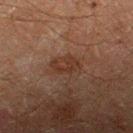Notes:
– workup · imaged on a skin check; not biopsied
– lighting · cross-polarized illumination
– imaging modality · total-body-photography crop, ~15 mm field of view
– subject · male, aged 58–62
– automated metrics · an area of roughly 3.5 mm², an eccentricity of roughly 0.85, and a symmetry-axis asymmetry near 0.55; a border-irregularity rating of about 7/10, a color-variation rating of about 0/10, and peripheral color asymmetry of about 0; a classifier nevus-likeness of about 15/100
– site · the right thigh
– size · ~3 mm (longest diameter)